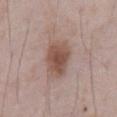follow-up: no biopsy performed (imaged during a skin exam)
location: the abdomen
tile lighting: white-light
image source: ~15 mm crop, total-body skin-cancer survey
lesion size: ~4.5 mm (longest diameter)
subject: male, aged 53 to 57
image-analysis metrics: an area of roughly 12 mm², an eccentricity of roughly 0.65, and a shape-asymmetry score of about 0.2 (0 = symmetric); border irregularity of about 2 on a 0–10 scale, a within-lesion color-variation index near 3.5/10, and peripheral color asymmetry of about 1